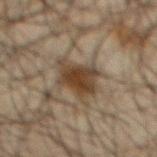This lesion was catalogued during total-body skin photography and was not selected for biopsy.
A male patient in their mid- to late 60s.
The lesion-visualizer software estimated a mean CIELAB color near L≈33 a*≈11 b*≈24 and a lesion–skin lightness drop of about 10. The analysis additionally found a border-irregularity rating of about 4/10 and a peripheral color-asymmetry measure near 1. The software also gave a classifier nevus-likeness of about 90/100.
From the chest.
A 15 mm close-up tile from a total-body photography series done for melanoma screening.